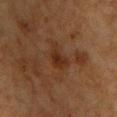Recorded during total-body skin imaging; not selected for excision or biopsy.
A 15 mm crop from a total-body photograph taken for skin-cancer surveillance.
A male patient aged approximately 60.
The tile uses cross-polarized illumination.
The total-body-photography lesion software estimated a symmetry-axis asymmetry near 0.4. The software also gave a lesion color around L≈25 a*≈18 b*≈26 in CIELAB and a normalized border contrast of about 7. And it measured border irregularity of about 4.5 on a 0–10 scale, internal color variation of about 3 on a 0–10 scale, and radial color variation of about 1. It also reported a nevus-likeness score of about 0/100 and a lesion-detection confidence of about 100/100.
Approximately 3.5 mm at its widest.
Located on the chest.